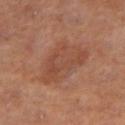follow-up = no biopsy performed (imaged during a skin exam); illumination = cross-polarized illumination; lesion diameter = ~7 mm (longest diameter); subject = female, aged around 55; acquisition = 15 mm crop, total-body photography; site = the right thigh; TBP lesion metrics = a lesion-detection confidence of about 100/100.